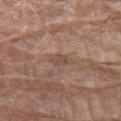Part of a total-body skin-imaging series; this lesion was reviewed on a skin check and was not flagged for biopsy. The lesion-visualizer software estimated a footprint of about 3.5 mm², a shape eccentricity near 0.85, and a shape-asymmetry score of about 0.3 (0 = symmetric). The analysis additionally found a lesion color around L≈49 a*≈17 b*≈26 in CIELAB, about 7 CIELAB-L* units darker than the surrounding skin, and a normalized border contrast of about 5.5. The analysis additionally found border irregularity of about 3.5 on a 0–10 scale, a color-variation rating of about 2.5/10, and a peripheral color-asymmetry measure near 1. And it measured a classifier nevus-likeness of about 0/100 and a detector confidence of about 80 out of 100 that the crop contains a lesion. On the front of the torso. The tile uses white-light illumination. The patient is a male approximately 80 years of age. Cropped from a total-body skin-imaging series; the visible field is about 15 mm. About 3 mm across.